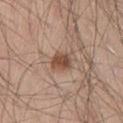Notes:
- anatomic site · the left thigh
- lesion diameter · about 2.5 mm
- patient · male, in their 30s
- automated lesion analysis · a lesion area of about 5 mm², an eccentricity of roughly 0.7, and a symmetry-axis asymmetry near 0.15; an automated nevus-likeness rating near 90 out of 100 and a detector confidence of about 100 out of 100 that the crop contains a lesion
- image · ~15 mm tile from a whole-body skin photo
- illumination · white-light